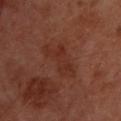  biopsy_status: not biopsied; imaged during a skin examination
  automated_metrics:
    area_mm2_approx: 9.0
    shape_asymmetry: 0.5
    cielab_L: 30
    cielab_a: 23
    cielab_b: 27
    vs_skin_contrast_norm: 5.5
    border_irregularity_0_10: 6.5
    color_variation_0_10: 3.0
    nevus_likeness_0_100: 0
    lesion_detection_confidence_0_100: 100
  lesion_size:
    long_diameter_mm_approx: 6.0
  site: upper back
  patient:
    sex: male
    age_approx: 50
  image:
    source: total-body photography crop
    field_of_view_mm: 15